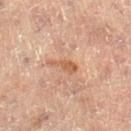{"biopsy_status": "not biopsied; imaged during a skin examination", "image": {"source": "total-body photography crop", "field_of_view_mm": 15}, "site": "leg", "patient": {"sex": "female", "age_approx": 80}, "automated_metrics": {"vs_skin_darker_L": 9.0, "vs_skin_contrast_norm": 7.0}, "lesion_size": {"long_diameter_mm_approx": 3.5}, "lighting": "cross-polarized"}